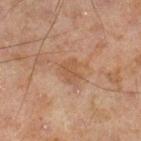Q: Lesion location?
A: the right lower leg
Q: What are the patient's age and sex?
A: male, in their mid-40s
Q: How was this image acquired?
A: ~15 mm tile from a whole-body skin photo